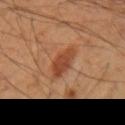Notes:
• notes · no biopsy performed (imaged during a skin exam)
• subject · male, aged 48 to 52
• image-analysis metrics · a lesion area of about 7.5 mm² and a symmetry-axis asymmetry near 0.25; a mean CIELAB color near L≈40 a*≈23 b*≈31, roughly 9 lightness units darker than nearby skin, and a lesion-to-skin contrast of about 7.5 (normalized; higher = more distinct); a nevus-likeness score of about 90/100 and a lesion-detection confidence of about 100/100
• acquisition · 15 mm crop, total-body photography
• body site · the right upper arm